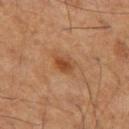The lesion was tiled from a total-body skin photograph and was not biopsied.
A close-up tile cropped from a whole-body skin photograph, about 15 mm across.
Located on the right thigh.
Automated tile analysis of the lesion measured a footprint of about 4 mm² and a symmetry-axis asymmetry near 0.25. The analysis additionally found an average lesion color of about L≈42 a*≈21 b*≈33 (CIELAB), about 9 CIELAB-L* units darker than the surrounding skin, and a normalized border contrast of about 7.5. It also reported a detector confidence of about 100 out of 100 that the crop contains a lesion.
A male patient in their 60s.
Measured at roughly 3 mm in maximum diameter.